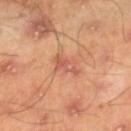workup: catalogued during a skin exam; not biopsied
patient: male, aged 43–47
body site: the right lower leg
illumination: cross-polarized illumination
lesion size: ≈3.5 mm
acquisition: ~15 mm tile from a whole-body skin photo